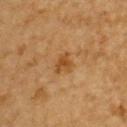| feature | finding |
|---|---|
| notes | no biopsy performed (imaged during a skin exam) |
| size | about 2.5 mm |
| subject | male, about 85 years old or older |
| tile lighting | cross-polarized |
| imaging modality | ~15 mm tile from a whole-body skin photo |
| site | the back |